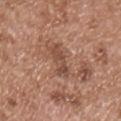Approximately 3.5 mm at its widest. A 15 mm crop from a total-body photograph taken for skin-cancer surveillance. Imaged with white-light lighting. A male subject roughly 55 years of age. Located on the chest. Automated image analysis of the tile measured an automated nevus-likeness rating near 0 out of 100.